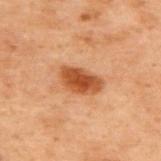Image and clinical context:
A 15 mm close-up extracted from a 3D total-body photography capture. The lesion is on the upper back. The patient is a male aged approximately 70.